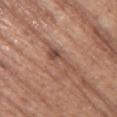No biopsy was performed on this lesion — it was imaged during a full skin examination and was not determined to be concerning. Cropped from a total-body skin-imaging series; the visible field is about 15 mm. Located on the right upper arm. The tile uses white-light illumination. Longest diameter approximately 4.5 mm. The lesion-visualizer software estimated a lesion area of about 5 mm², a shape eccentricity near 0.95, and a shape-asymmetry score of about 0.6 (0 = symmetric). It also reported a mean CIELAB color near L≈49 a*≈21 b*≈27, a lesion–skin lightness drop of about 10, and a lesion-to-skin contrast of about 7 (normalized; higher = more distinct). It also reported a nevus-likeness score of about 15/100 and a detector confidence of about 95 out of 100 that the crop contains a lesion. A female subject, aged approximately 65.